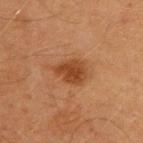Impression:
Captured during whole-body skin photography for melanoma surveillance; the lesion was not biopsied.
Background:
This is a cross-polarized tile. The lesion is on the upper back. The subject is a male about 45 years old. An algorithmic analysis of the crop reported a lesion color around L≈36 a*≈22 b*≈32 in CIELAB and a normalized border contrast of about 8.5. The analysis additionally found a classifier nevus-likeness of about 90/100 and a lesion-detection confidence of about 100/100. This image is a 15 mm lesion crop taken from a total-body photograph.